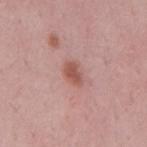Findings:
– lesion diameter · about 3 mm
– acquisition · ~15 mm tile from a whole-body skin photo
– lighting · white-light illumination
– location · the mid back
– subject · male, roughly 50 years of age